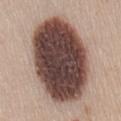workup: imaged on a skin check; not biopsied | image: ~15 mm crop, total-body skin-cancer survey | subject: female, roughly 50 years of age | lighting: white-light illumination | diameter: ~11 mm (longest diameter) | site: the mid back | automated metrics: a lesion-to-skin contrast of about 17 (normalized; higher = more distinct); a border-irregularity index near 1/10, internal color variation of about 8 on a 0–10 scale, and a peripheral color-asymmetry measure near 2.